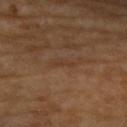Q: Was this lesion biopsied?
A: catalogued during a skin exam; not biopsied
Q: Lesion location?
A: the left upper arm
Q: How large is the lesion?
A: about 3 mm
Q: Who is the patient?
A: female, roughly 70 years of age
Q: What is the imaging modality?
A: ~15 mm tile from a whole-body skin photo